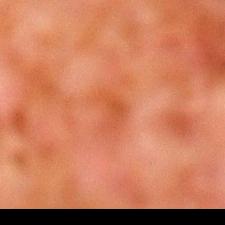<case>
<biopsy_status>not biopsied; imaged during a skin examination</biopsy_status>
<image>
  <source>total-body photography crop</source>
  <field_of_view_mm>15</field_of_view_mm>
</image>
<automated_metrics>
  <cielab_L>42</cielab_L>
  <cielab_a>28</cielab_a>
  <cielab_b>35</cielab_b>
  <vs_skin_contrast_norm>5.5</vs_skin_contrast_norm>
  <border_irregularity_0_10>4.0</border_irregularity_0_10>
  <color_variation_0_10>3.0</color_variation_0_10>
  <peripheral_color_asymmetry>1.0</peripheral_color_asymmetry>
</automated_metrics>
<site>left lower leg</site>
<patient>
  <sex>male</sex>
  <age_approx>80</age_approx>
</patient>
<lighting>cross-polarized</lighting>
<lesion_size>
  <long_diameter_mm_approx>3.5</long_diameter_mm_approx>
</lesion_size>
</case>